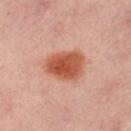Assessment:
The lesion was tiled from a total-body skin photograph and was not biopsied.
Image and clinical context:
A female patient, aged 48 to 52. A roughly 15 mm field-of-view crop from a total-body skin photograph. About 5 mm across. Captured under cross-polarized illumination. The lesion is located on the leg.